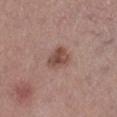Assessment:
Recorded during total-body skin imaging; not selected for excision or biopsy.
Clinical summary:
The lesion-visualizer software estimated a lesion area of about 6 mm², a shape eccentricity near 0.65, and a symmetry-axis asymmetry near 0.25. And it measured border irregularity of about 2.5 on a 0–10 scale, a color-variation rating of about 3.5/10, and peripheral color asymmetry of about 1. The software also gave an automated nevus-likeness rating near 60 out of 100 and a detector confidence of about 100 out of 100 that the crop contains a lesion. The lesion is on the leg. Cropped from a whole-body photographic skin survey; the tile spans about 15 mm. A female patient, about 60 years old. The tile uses white-light illumination.